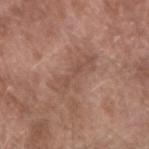Context: The total-body-photography lesion software estimated a footprint of about 12 mm², an outline eccentricity of about 0.95 (0 = round, 1 = elongated), and a symmetry-axis asymmetry near 0.35. It also reported a border-irregularity rating of about 5/10 and peripheral color asymmetry of about 1. From the right upper arm. A male patient about 55 years old. A roughly 15 mm field-of-view crop from a total-body skin photograph. The tile uses white-light illumination. Measured at roughly 6.5 mm in maximum diameter.